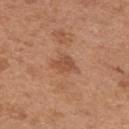{
  "biopsy_status": "not biopsied; imaged during a skin examination"
}